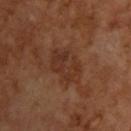Q: Patient demographics?
A: male, in their mid-60s
Q: Automated lesion metrics?
A: an average lesion color of about L≈31 a*≈20 b*≈27 (CIELAB), about 6 CIELAB-L* units darker than the surrounding skin, and a lesion-to-skin contrast of about 6 (normalized; higher = more distinct); a color-variation rating of about 4.5/10
Q: What kind of image is this?
A: 15 mm crop, total-body photography
Q: Illumination type?
A: cross-polarized
Q: How large is the lesion?
A: about 4.5 mm
Q: What is the anatomic site?
A: the upper back